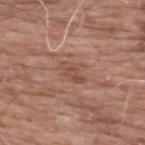Clinical impression: Part of a total-body skin-imaging series; this lesion was reviewed on a skin check and was not flagged for biopsy. Clinical summary: A male subject, roughly 60 years of age. Located on the upper back. A close-up tile cropped from a whole-body skin photograph, about 15 mm across. The lesion-visualizer software estimated an outline eccentricity of about 0.75 (0 = round, 1 = elongated) and a shape-asymmetry score of about 0.3 (0 = symmetric). It also reported an average lesion color of about L≈49 a*≈21 b*≈27 (CIELAB), a lesion–skin lightness drop of about 7, and a lesion-to-skin contrast of about 5.5 (normalized; higher = more distinct). The software also gave an automated nevus-likeness rating near 0 out of 100 and a detector confidence of about 100 out of 100 that the crop contains a lesion. Measured at roughly 3 mm in maximum diameter. Imaged with white-light lighting.